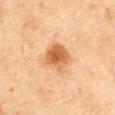• follow-up · no biopsy performed (imaged during a skin exam)
• body site · the mid back
• lesion size · about 4 mm
• patient · male, aged 43–47
• image · total-body-photography crop, ~15 mm field of view
• tile lighting · cross-polarized illumination